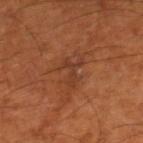Impression:
Recorded during total-body skin imaging; not selected for excision or biopsy.
Acquisition and patient details:
The tile uses cross-polarized illumination. A roughly 15 mm field-of-view crop from a total-body skin photograph. From the left leg. A male subject aged 63 to 67. Automated image analysis of the tile measured an area of roughly 5 mm², an eccentricity of roughly 0.7, and two-axis asymmetry of about 0.55. The analysis additionally found a mean CIELAB color near L≈37 a*≈24 b*≈31, a lesion–skin lightness drop of about 6, and a lesion-to-skin contrast of about 5.5 (normalized; higher = more distinct).